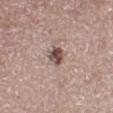<record>
<biopsy_status>not biopsied; imaged during a skin examination</biopsy_status>
<site>left lower leg</site>
<image>
  <source>total-body photography crop</source>
  <field_of_view_mm>15</field_of_view_mm>
</image>
<patient>
  <sex>male</sex>
  <age_approx>65</age_approx>
</patient>
<lesion_size>
  <long_diameter_mm_approx>2.5</long_diameter_mm_approx>
</lesion_size>
<lighting>white-light</lighting>
<automated_metrics>
  <cielab_L>48</cielab_L>
  <cielab_a>17</cielab_a>
  <cielab_b>20</cielab_b>
  <vs_skin_darker_L>16.0</vs_skin_darker_L>
  <vs_skin_contrast_norm>11.0</vs_skin_contrast_norm>
  <border_irregularity_0_10>2.0</border_irregularity_0_10>
  <nevus_likeness_0_100>80</nevus_likeness_0_100>
  <lesion_detection_confidence_0_100>100</lesion_detection_confidence_0_100>
</automated_metrics>
</record>